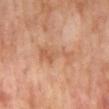This lesion was catalogued during total-body skin photography and was not selected for biopsy.
Cropped from a total-body skin-imaging series; the visible field is about 15 mm.
The patient is a male aged around 65.
Imaged with cross-polarized lighting.
Located on the mid back.
An algorithmic analysis of the crop reported an outline eccentricity of about 0.95 (0 = round, 1 = elongated). The analysis additionally found a border-irregularity index near 7/10, a color-variation rating of about 3/10, and peripheral color asymmetry of about 1.